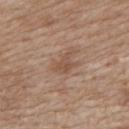Q: Was this lesion biopsied?
A: total-body-photography surveillance lesion; no biopsy
Q: What is the imaging modality?
A: total-body-photography crop, ~15 mm field of view
Q: Patient demographics?
A: female, aged approximately 40
Q: Where on the body is the lesion?
A: the upper back